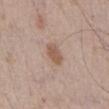Clinical impression:
Imaged during a routine full-body skin examination; the lesion was not biopsied and no histopathology is available.
Clinical summary:
A male patient roughly 65 years of age. A 15 mm crop from a total-body photograph taken for skin-cancer surveillance. From the front of the torso.